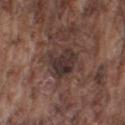Imaged during a routine full-body skin examination; the lesion was not biopsied and no histopathology is available. The patient is a male aged approximately 75. A lesion tile, about 15 mm wide, cut from a 3D total-body photograph. On the left upper arm.